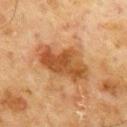notes = catalogued during a skin exam; not biopsied | acquisition = ~15 mm crop, total-body skin-cancer survey | lesion diameter = about 7 mm | location = the mid back | tile lighting = cross-polarized illumination | subject = male, aged 68–72.